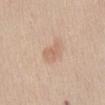biopsy status=imaged on a skin check; not biopsied
imaging modality=~15 mm crop, total-body skin-cancer survey
subject=female, approximately 40 years of age
diameter=~3 mm (longest diameter)
lighting=white-light illumination
site=the abdomen